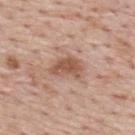follow-up: imaged on a skin check; not biopsied
acquisition: ~15 mm tile from a whole-body skin photo
automated lesion analysis: a border-irregularity index near 3.5/10, a within-lesion color-variation index near 2.5/10, and a peripheral color-asymmetry measure near 1; an automated nevus-likeness rating near 70 out of 100
subject: male, aged approximately 75
lighting: white-light illumination
lesion diameter: ≈4 mm
anatomic site: the upper back